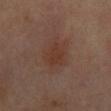| field | value |
|---|---|
| biopsy status | no biopsy performed (imaged during a skin exam) |